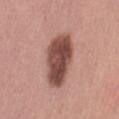Findings:
- image — ~15 mm tile from a whole-body skin photo
- subject — female, aged approximately 30
- TBP lesion metrics — a lesion color around L≈48 a*≈23 b*≈24 in CIELAB, a lesion–skin lightness drop of about 17, and a normalized border contrast of about 11.5; a color-variation rating of about 5.5/10 and a peripheral color-asymmetry measure near 2; a lesion-detection confidence of about 100/100
- lesion diameter — ~6.5 mm (longest diameter)
- lighting — white-light
- site — the left thigh